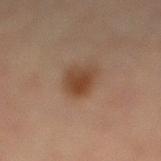| feature | finding |
|---|---|
| follow-up | imaged on a skin check; not biopsied |
| acquisition | total-body-photography crop, ~15 mm field of view |
| subject | female, approximately 70 years of age |
| body site | the right lower leg |
| lesion diameter | about 3.5 mm |